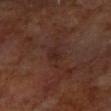Recorded during total-body skin imaging; not selected for excision or biopsy. Cropped from a total-body skin-imaging series; the visible field is about 15 mm. Automated image analysis of the tile measured an average lesion color of about L≈25 a*≈19 b*≈21 (CIELAB) and roughly 5 lightness units darker than nearby skin. A female patient in their mid-60s. The lesion is on the arm. The lesion's longest dimension is about 4 mm. Imaged with cross-polarized lighting.